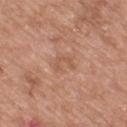{
  "biopsy_status": "not biopsied; imaged during a skin examination",
  "patient": {
    "sex": "male",
    "age_approx": 50
  },
  "image": {
    "source": "total-body photography crop",
    "field_of_view_mm": 15
  },
  "site": "back"
}